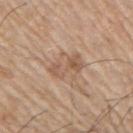Clinical impression:
The lesion was tiled from a total-body skin photograph and was not biopsied.
Image and clinical context:
Imaged with white-light lighting. Longest diameter approximately 4 mm. From the right upper arm. The patient is a male in their mid-60s. A 15 mm crop from a total-body photograph taken for skin-cancer surveillance.